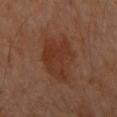Imaged during a routine full-body skin examination; the lesion was not biopsied and no histopathology is available. Captured under cross-polarized illumination. The lesion is located on the left arm. The lesion's longest dimension is about 6 mm. A female subject in their 60s. A 15 mm close-up extracted from a 3D total-body photography capture.